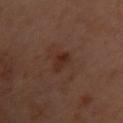Assessment:
Captured during whole-body skin photography for melanoma surveillance; the lesion was not biopsied.
Clinical summary:
A female subject aged around 55. From the front of the torso. This is a cross-polarized tile. A region of skin cropped from a whole-body photographic capture, roughly 15 mm wide.